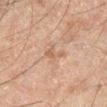This lesion was catalogued during total-body skin photography and was not selected for biopsy. Automated image analysis of the tile measured a shape-asymmetry score of about 0.5 (0 = symmetric). It also reported a lesion color around L≈48 a*≈16 b*≈27 in CIELAB, roughly 6 lightness units darker than nearby skin, and a normalized lesion–skin contrast near 5.5. It also reported a border-irregularity rating of about 6/10, internal color variation of about 0 on a 0–10 scale, and peripheral color asymmetry of about 0. And it measured an automated nevus-likeness rating near 0 out of 100 and a lesion-detection confidence of about 100/100. On the leg. The patient is a male about 45 years old. Cropped from a whole-body photographic skin survey; the tile spans about 15 mm. Captured under cross-polarized illumination.